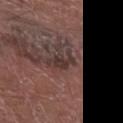Findings:
* follow-up — catalogued during a skin exam; not biopsied
* anatomic site — the left forearm
* TBP lesion metrics — a lesion area of about 5 mm² and two-axis asymmetry of about 0.45; a border-irregularity rating of about 7/10 and peripheral color asymmetry of about 1
* lighting — white-light
* patient — male, aged around 70
* acquisition — 15 mm crop, total-body photography